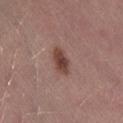biopsy status: total-body-photography surveillance lesion; no biopsy
location: the right thigh
subject: female, approximately 40 years of age
lighting: white-light illumination
image: 15 mm crop, total-body photography